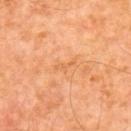The lesion was tiled from a total-body skin photograph and was not biopsied. Approximately 3 mm at its widest. A region of skin cropped from a whole-body photographic capture, roughly 15 mm wide. The patient is a male aged around 55. The tile uses cross-polarized illumination. The total-body-photography lesion software estimated a footprint of about 2.5 mm², a shape eccentricity near 0.95, and two-axis asymmetry of about 0.4. It also reported a border-irregularity rating of about 5/10, a within-lesion color-variation index near 0/10, and peripheral color asymmetry of about 0. And it measured an automated nevus-likeness rating near 0 out of 100. Located on the back.